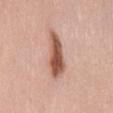The lesion was tiled from a total-body skin photograph and was not biopsied. The lesion is on the mid back. The patient is a female in their mid-40s. A 15 mm crop from a total-body photograph taken for skin-cancer surveillance. Imaged with white-light lighting.